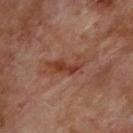Recorded during total-body skin imaging; not selected for excision or biopsy. A region of skin cropped from a whole-body photographic capture, roughly 15 mm wide. The lesion is on the upper back. A male subject, in their 70s.